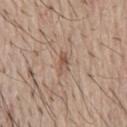Impression:
No biopsy was performed on this lesion — it was imaged during a full skin examination and was not determined to be concerning.
Acquisition and patient details:
This is a white-light tile. Longest diameter approximately 2.5 mm. The patient is a male in their mid- to late 60s. On the chest. The total-body-photography lesion software estimated a classifier nevus-likeness of about 0/100 and a lesion-detection confidence of about 100/100. Cropped from a total-body skin-imaging series; the visible field is about 15 mm.